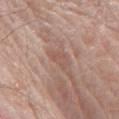Part of a total-body skin-imaging series; this lesion was reviewed on a skin check and was not flagged for biopsy.
On the front of the torso.
The total-body-photography lesion software estimated a border-irregularity index near 4/10, a color-variation rating of about 1/10, and a peripheral color-asymmetry measure near 0.5.
The subject is a male approximately 80 years of age.
This is a white-light tile.
A close-up tile cropped from a whole-body skin photograph, about 15 mm across.
Approximately 3 mm at its widest.